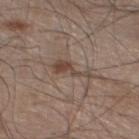The lesion was tiled from a total-body skin photograph and was not biopsied. This is a white-light tile. About 5 mm across. Cropped from a whole-body photographic skin survey; the tile spans about 15 mm. A male subject approximately 60 years of age. The lesion is on the left lower leg.